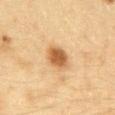The patient is a female roughly 30 years of age.
A close-up tile cropped from a whole-body skin photograph, about 15 mm across.
This is a cross-polarized tile.
From the front of the torso.
The total-body-photography lesion software estimated a mean CIELAB color near L≈54 a*≈20 b*≈39, roughly 14 lightness units darker than nearby skin, and a normalized border contrast of about 9.5. It also reported a color-variation rating of about 3.5/10. And it measured a classifier nevus-likeness of about 100/100 and lesion-presence confidence of about 100/100.